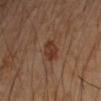| key | value |
|---|---|
| follow-up | catalogued during a skin exam; not biopsied |
| diameter | ≈3 mm |
| imaging modality | total-body-photography crop, ~15 mm field of view |
| anatomic site | the left forearm |
| TBP lesion metrics | an area of roughly 4.5 mm²; a color-variation rating of about 2/10 and a peripheral color-asymmetry measure near 1; a detector confidence of about 100 out of 100 that the crop contains a lesion |
| subject | female, roughly 45 years of age |